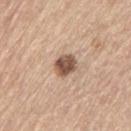Q: Was this lesion biopsied?
A: total-body-photography surveillance lesion; no biopsy
Q: Where on the body is the lesion?
A: the left thigh
Q: Automated lesion metrics?
A: a shape-asymmetry score of about 0.15 (0 = symmetric); a lesion color around L≈53 a*≈18 b*≈28 in CIELAB, roughly 17 lightness units darker than nearby skin, and a normalized border contrast of about 11; a border-irregularity rating of about 1.5/10, a within-lesion color-variation index near 5/10, and radial color variation of about 1.5; a classifier nevus-likeness of about 85/100 and a detector confidence of about 100 out of 100 that the crop contains a lesion
Q: Who is the patient?
A: male, about 70 years old
Q: How large is the lesion?
A: ~3 mm (longest diameter)
Q: What kind of image is this?
A: ~15 mm tile from a whole-body skin photo